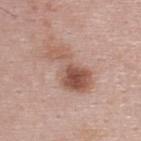Clinical summary:
A male subject, in their mid- to late 20s. Cropped from a whole-body photographic skin survey; the tile spans about 15 mm. The lesion is located on the back. Measured at roughly 6.5 mm in maximum diameter.